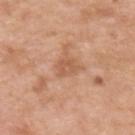biopsy status: catalogued during a skin exam; not biopsied | lesion diameter: about 3 mm | lighting: white-light | image: 15 mm crop, total-body photography | body site: the upper back | patient: male, roughly 50 years of age.